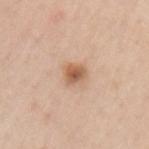biopsy status: no biopsy performed (imaged during a skin exam)
diameter: ~2.5 mm (longest diameter)
imaging modality: 15 mm crop, total-body photography
subject: male, aged approximately 60
location: the left upper arm
automated lesion analysis: a lesion color around L≈59 a*≈20 b*≈33 in CIELAB, a lesion–skin lightness drop of about 12, and a lesion-to-skin contrast of about 8.5 (normalized; higher = more distinct); a nevus-likeness score of about 95/100 and lesion-presence confidence of about 100/100